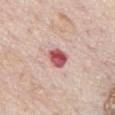Clinical impression:
Imaged during a routine full-body skin examination; the lesion was not biopsied and no histopathology is available.
Background:
Longest diameter approximately 3 mm. A male patient in their mid- to late 70s. An algorithmic analysis of the crop reported a lesion area of about 5 mm², an outline eccentricity of about 0.7 (0 = round, 1 = elongated), and two-axis asymmetry of about 0.2. It also reported internal color variation of about 5.5 on a 0–10 scale and a peripheral color-asymmetry measure near 1.5. And it measured an automated nevus-likeness rating near 0 out of 100 and a detector confidence of about 100 out of 100 that the crop contains a lesion. From the chest. A 15 mm close-up tile from a total-body photography series done for melanoma screening. The tile uses white-light illumination.